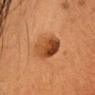Q: Is there a histopathology result?
A: total-body-photography surveillance lesion; no biopsy
Q: How was the tile lit?
A: cross-polarized
Q: What is the imaging modality?
A: 15 mm crop, total-body photography
Q: Where on the body is the lesion?
A: the head or neck
Q: How large is the lesion?
A: about 5 mm
Q: What are the patient's age and sex?
A: female, aged approximately 30